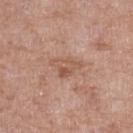{"biopsy_status": "not biopsied; imaged during a skin examination", "site": "left lower leg", "lighting": "white-light", "patient": {"sex": "female", "age_approx": 75}, "image": {"source": "total-body photography crop", "field_of_view_mm": 15}, "lesion_size": {"long_diameter_mm_approx": 4.0}}